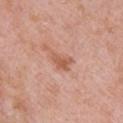Q: Was this lesion biopsied?
A: total-body-photography surveillance lesion; no biopsy
Q: What is the anatomic site?
A: the front of the torso
Q: How was this image acquired?
A: ~15 mm crop, total-body skin-cancer survey
Q: Who is the patient?
A: female, about 40 years old
Q: What is the lesion's diameter?
A: ≈3 mm
Q: What did automated image analysis measure?
A: a lesion area of about 4.5 mm², an eccentricity of roughly 0.75, and a shape-asymmetry score of about 0.4 (0 = symmetric); roughly 9 lightness units darker than nearby skin; a border-irregularity index near 4/10, internal color variation of about 2.5 on a 0–10 scale, and a peripheral color-asymmetry measure near 1; a classifier nevus-likeness of about 10/100 and a detector confidence of about 100 out of 100 that the crop contains a lesion
Q: What lighting was used for the tile?
A: white-light illumination